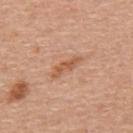This lesion was catalogued during total-body skin photography and was not selected for biopsy.
The lesion-visualizer software estimated an average lesion color of about L≈57 a*≈23 b*≈34 (CIELAB) and a lesion-to-skin contrast of about 6.5 (normalized; higher = more distinct). It also reported a border-irregularity rating of about 4/10.
Imaged with white-light lighting.
Located on the upper back.
A close-up tile cropped from a whole-body skin photograph, about 15 mm across.
A male subject, aged approximately 55.